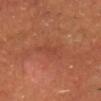Impression:
The lesion was photographed on a routine skin check and not biopsied; there is no pathology result.
Clinical summary:
A close-up tile cropped from a whole-body skin photograph, about 15 mm across. The recorded lesion diameter is about 4.5 mm. Automated tile analysis of the lesion measured an area of roughly 7.5 mm² and an outline eccentricity of about 0.9 (0 = round, 1 = elongated). Captured under cross-polarized illumination. The lesion is located on the head or neck. A male patient in their mid-50s.